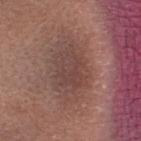Cropped from a total-body skin-imaging series; the visible field is about 15 mm. The lesion is located on the head or neck. A female patient in their mid-20s. Automated tile analysis of the lesion measured a shape eccentricity near 0.6. It also reported a normalized lesion–skin contrast near 6. The analysis additionally found a within-lesion color-variation index near 3/10 and peripheral color asymmetry of about 1. The software also gave a classifier nevus-likeness of about 5/100 and lesion-presence confidence of about 95/100. Imaged with white-light lighting.